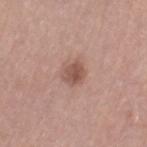No biopsy was performed on this lesion — it was imaged during a full skin examination and was not determined to be concerning.
The subject is a female aged 63–67.
An algorithmic analysis of the crop reported a shape eccentricity near 0.6 and a shape-asymmetry score of about 0.25 (0 = symmetric). It also reported a mean CIELAB color near L≈52 a*≈21 b*≈25, about 11 CIELAB-L* units darker than the surrounding skin, and a lesion-to-skin contrast of about 7.5 (normalized; higher = more distinct). The software also gave a border-irregularity rating of about 2.5/10, a color-variation rating of about 3.5/10, and peripheral color asymmetry of about 1. The analysis additionally found an automated nevus-likeness rating near 50 out of 100 and a lesion-detection confidence of about 100/100.
A 15 mm close-up extracted from a 3D total-body photography capture.
On the leg.
Measured at roughly 3 mm in maximum diameter.